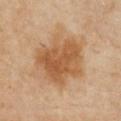notes: imaged on a skin check; not biopsied | size: about 6.5 mm | image source: ~15 mm crop, total-body skin-cancer survey | location: the chest | tile lighting: cross-polarized illumination | patient: female, aged 38 to 42.